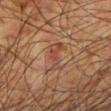Imaged during a routine full-body skin examination; the lesion was not biopsied and no histopathology is available. An algorithmic analysis of the crop reported a lesion area of about 6.5 mm² and a symmetry-axis asymmetry near 0.45. The tile uses cross-polarized illumination. A male subject, in their mid-70s. A roughly 15 mm field-of-view crop from a total-body skin photograph. From the right upper arm.